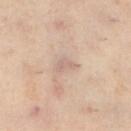Impression:
Imaged during a routine full-body skin examination; the lesion was not biopsied and no histopathology is available.
Background:
The tile uses cross-polarized illumination. Located on the leg. Automated tile analysis of the lesion measured a footprint of about 2.5 mm², an outline eccentricity of about 0.9 (0 = round, 1 = elongated), and a symmetry-axis asymmetry near 0.5. The analysis additionally found an average lesion color of about L≈56 a*≈14 b*≈22 (CIELAB), a lesion–skin lightness drop of about 6, and a normalized border contrast of about 5. Cropped from a whole-body photographic skin survey; the tile spans about 15 mm. About 2.5 mm across. The subject is a female approximately 60 years of age.